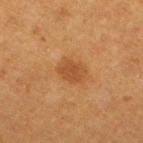The lesion was photographed on a routine skin check and not biopsied; there is no pathology result. A 15 mm close-up extracted from a 3D total-body photography capture. A female patient aged 38–42. The tile uses cross-polarized illumination. About 3 mm across. The lesion is located on the leg.